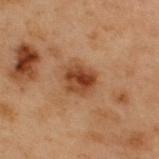<record>
  <biopsy_status>not biopsied; imaged during a skin examination</biopsy_status>
  <site>upper back</site>
  <patient>
    <sex>male</sex>
    <age_approx>55</age_approx>
  </patient>
  <image>
    <source>total-body photography crop</source>
    <field_of_view_mm>15</field_of_view_mm>
  </image>
</record>